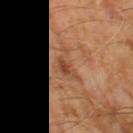Imaged during a routine full-body skin examination; the lesion was not biopsied and no histopathology is available.
Cropped from a whole-body photographic skin survey; the tile spans about 15 mm.
The lesion-visualizer software estimated an area of roughly 5.5 mm², an eccentricity of roughly 0.9, and two-axis asymmetry of about 0.55. It also reported a border-irregularity index near 6.5/10 and peripheral color asymmetry of about 0.5.
The patient is a male in their mid- to late 60s.